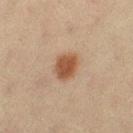| key | value |
|---|---|
| follow-up | catalogued during a skin exam; not biopsied |
| tile lighting | cross-polarized |
| image-analysis metrics | an area of roughly 7.5 mm² and an eccentricity of roughly 0.7; an average lesion color of about L≈45 a*≈18 b*≈30 (CIELAB), roughly 11 lightness units darker than nearby skin, and a lesion-to-skin contrast of about 9 (normalized; higher = more distinct); a border-irregularity rating of about 2.5/10 and internal color variation of about 3 on a 0–10 scale; a nevus-likeness score of about 100/100 and lesion-presence confidence of about 100/100 |
| patient | female, in their 20s |
| imaging modality | ~15 mm tile from a whole-body skin photo |
| site | the left thigh |